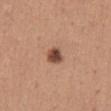Q: Was a biopsy performed?
A: imaged on a skin check; not biopsied
Q: What kind of image is this?
A: 15 mm crop, total-body photography
Q: Lesion location?
A: the chest
Q: Who is the patient?
A: female, in their mid-40s